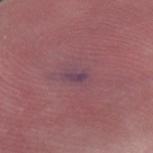Findings:
- notes: catalogued during a skin exam; not biopsied
- patient: male, about 55 years old
- image: 15 mm crop, total-body photography
- diameter: about 3 mm
- body site: the right forearm
- illumination: white-light